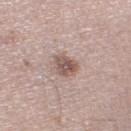This is a white-light tile.
On the left thigh.
A female patient in their 50s.
Cropped from a total-body skin-imaging series; the visible field is about 15 mm.